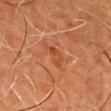biopsy status: imaged on a skin check; not biopsied | subject: male, approximately 60 years of age | site: the head or neck | automated metrics: a lesion area of about 3.5 mm² and two-axis asymmetry of about 0.4; an automated nevus-likeness rating near 0 out of 100 and lesion-presence confidence of about 100/100 | image: ~15 mm tile from a whole-body skin photo.